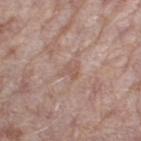This lesion was catalogued during total-body skin photography and was not selected for biopsy.